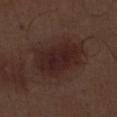Notes:
* follow-up: no biopsy performed (imaged during a skin exam)
* image: total-body-photography crop, ~15 mm field of view
* tile lighting: white-light
* subject: male, aged approximately 70
* body site: the leg
* lesion diameter: ~6.5 mm (longest diameter)
* automated lesion analysis: an outline eccentricity of about 0.75 (0 = round, 1 = elongated) and two-axis asymmetry of about 0.2; a mean CIELAB color near L≈23 a*≈18 b*≈19, about 7 CIELAB-L* units darker than the surrounding skin, and a lesion-to-skin contrast of about 8.5 (normalized; higher = more distinct); a within-lesion color-variation index near 3/10